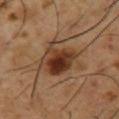{
  "site": "chest",
  "automated_metrics": {
    "cielab_L": 37,
    "cielab_a": 18,
    "cielab_b": 29,
    "vs_skin_darker_L": 11.0,
    "vs_skin_contrast_norm": 9.0,
    "color_variation_0_10": 9.0,
    "peripheral_color_asymmetry": 2.5,
    "lesion_detection_confidence_0_100": 100
  },
  "lighting": "cross-polarized",
  "patient": {
    "sex": "male",
    "age_approx": 55
  },
  "lesion_size": {
    "long_diameter_mm_approx": 6.0
  },
  "image": {
    "source": "total-body photography crop",
    "field_of_view_mm": 15
  }
}